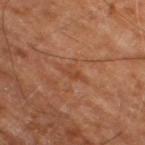Case summary:
• follow-up: no biopsy performed (imaged during a skin exam)
• acquisition: 15 mm crop, total-body photography
• lesion diameter: about 3 mm
• site: the right thigh
• subject: in their mid-60s
• illumination: cross-polarized